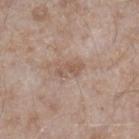biopsy_status: not biopsied; imaged during a skin examination
image:
  source: total-body photography crop
  field_of_view_mm: 15
patient:
  sex: male
  age_approx: 45
lesion_size:
  long_diameter_mm_approx: 3.5
site: right lower leg